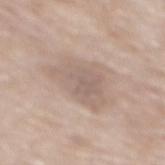Assessment:
Part of a total-body skin-imaging series; this lesion was reviewed on a skin check and was not flagged for biopsy.
Image and clinical context:
This is a white-light tile. A roughly 15 mm field-of-view crop from a total-body skin photograph. Automated image analysis of the tile measured a shape eccentricity near 0.8 and a symmetry-axis asymmetry near 0.35. The analysis additionally found border irregularity of about 4.5 on a 0–10 scale, a within-lesion color-variation index near 2.5/10, and radial color variation of about 0.5. The software also gave a classifier nevus-likeness of about 0/100 and lesion-presence confidence of about 90/100. The recorded lesion diameter is about 6 mm. Located on the back. The patient is a female in their mid-70s.